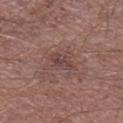Clinical impression: Imaged during a routine full-body skin examination; the lesion was not biopsied and no histopathology is available. Clinical summary: Located on the leg. Measured at roughly 3 mm in maximum diameter. Cropped from a total-body skin-imaging series; the visible field is about 15 mm. The tile uses white-light illumination. The subject is a male aged around 70.